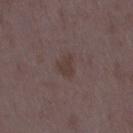follow-up: no biopsy performed (imaged during a skin exam)
image: ~15 mm crop, total-body skin-cancer survey
anatomic site: the right thigh
subject: female, roughly 35 years of age
size: ≈2.5 mm
lighting: white-light illumination
automated lesion analysis: an average lesion color of about L≈37 a*≈14 b*≈19 (CIELAB), roughly 6 lightness units darker than nearby skin, and a lesion-to-skin contrast of about 6.5 (normalized; higher = more distinct); a peripheral color-asymmetry measure near 0.5; a nevus-likeness score of about 0/100 and lesion-presence confidence of about 100/100